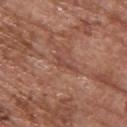Captured during whole-body skin photography for melanoma surveillance; the lesion was not biopsied.